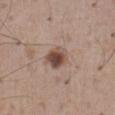biopsy status: imaged on a skin check; not biopsied
tile lighting: white-light illumination
subject: male, roughly 65 years of age
image source: total-body-photography crop, ~15 mm field of view
lesion size: about 3 mm
anatomic site: the abdomen
TBP lesion metrics: a lesion area of about 6.5 mm² and a symmetry-axis asymmetry near 0.15; a mean CIELAB color near L≈48 a*≈18 b*≈25, about 13 CIELAB-L* units darker than the surrounding skin, and a lesion-to-skin contrast of about 9.5 (normalized; higher = more distinct); an automated nevus-likeness rating near 95 out of 100